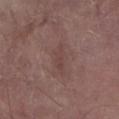Q: Was a biopsy performed?
A: no biopsy performed (imaged during a skin exam)
Q: Automated lesion metrics?
A: a footprint of about 4 mm², an outline eccentricity of about 0.9 (0 = round, 1 = elongated), and a shape-asymmetry score of about 0.4 (0 = symmetric); an average lesion color of about L≈42 a*≈18 b*≈20 (CIELAB) and a normalized lesion–skin contrast near 4.5
Q: Illumination type?
A: white-light illumination
Q: What is the imaging modality?
A: ~15 mm tile from a whole-body skin photo
Q: What are the patient's age and sex?
A: male, in their mid- to late 60s
Q: Where on the body is the lesion?
A: the left forearm
Q: What is the lesion's diameter?
A: ~3.5 mm (longest diameter)